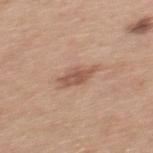Located on the mid back. A male subject, aged 28 to 32. The tile uses white-light illumination. Cropped from a whole-body photographic skin survey; the tile spans about 15 mm. About 4 mm across.